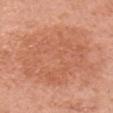<tbp_lesion>
  <biopsy_status>not biopsied; imaged during a skin examination</biopsy_status>
  <patient>
    <sex>female</sex>
    <age_approx>65</age_approx>
  </patient>
  <site>head or neck</site>
  <image>
    <source>total-body photography crop</source>
    <field_of_view_mm>15</field_of_view_mm>
  </image>
</tbp_lesion>